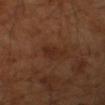biopsy_status: not biopsied; imaged during a skin examination
image:
  source: total-body photography crop
  field_of_view_mm: 15
lighting: cross-polarized
patient:
  sex: male
  age_approx: 65